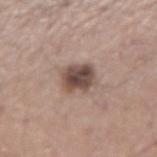Captured during whole-body skin photography for melanoma surveillance; the lesion was not biopsied. On the arm. This is a white-light tile. A lesion tile, about 15 mm wide, cut from a 3D total-body photograph. The patient is a male about 55 years old.